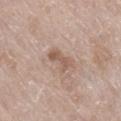No biopsy was performed on this lesion — it was imaged during a full skin examination and was not determined to be concerning.
The lesion is on the right lower leg.
The tile uses white-light illumination.
An algorithmic analysis of the crop reported two-axis asymmetry of about 0.4. The software also gave a lesion color around L≈57 a*≈17 b*≈27 in CIELAB and roughly 9 lightness units darker than nearby skin. And it measured border irregularity of about 4.5 on a 0–10 scale, a within-lesion color-variation index near 2.5/10, and peripheral color asymmetry of about 0.5. The software also gave an automated nevus-likeness rating near 0 out of 100 and a detector confidence of about 100 out of 100 that the crop contains a lesion.
A 15 mm close-up extracted from a 3D total-body photography capture.
A female patient aged 73 to 77.
Measured at roughly 3.5 mm in maximum diameter.